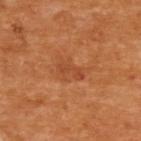Assessment:
This lesion was catalogued during total-body skin photography and was not selected for biopsy.
Context:
A female patient, roughly 55 years of age. Cropped from a total-body skin-imaging series; the visible field is about 15 mm. The lesion is located on the upper back. About 3 mm across. Automated tile analysis of the lesion measured an area of roughly 3 mm², a shape eccentricity near 0.95, and a symmetry-axis asymmetry near 0.4. The analysis additionally found a mean CIELAB color near L≈48 a*≈29 b*≈40, about 7 CIELAB-L* units darker than the surrounding skin, and a normalized border contrast of about 5.5. The software also gave a border-irregularity rating of about 5/10, a within-lesion color-variation index near 0/10, and radial color variation of about 0. Imaged with cross-polarized lighting.